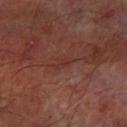<tbp_lesion>
<biopsy_status>not biopsied; imaged during a skin examination</biopsy_status>
<lesion_size>
  <long_diameter_mm_approx>2.5</long_diameter_mm_approx>
</lesion_size>
<patient>
  <sex>male</sex>
  <age_approx>65</age_approx>
</patient>
<site>left forearm</site>
<automated_metrics>
  <border_irregularity_0_10>4.0</border_irregularity_0_10>
  <nevus_likeness_0_100>0</nevus_likeness_0_100>
  <lesion_detection_confidence_0_100>50</lesion_detection_confidence_0_100>
</automated_metrics>
<image>
  <source>total-body photography crop</source>
  <field_of_view_mm>15</field_of_view_mm>
</image>
<lighting>cross-polarized</lighting>
</tbp_lesion>